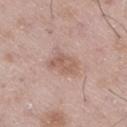Impression:
Part of a total-body skin-imaging series; this lesion was reviewed on a skin check and was not flagged for biopsy.
Background:
The total-body-photography lesion software estimated an area of roughly 7 mm² and a shape-asymmetry score of about 0.2 (0 = symmetric). It also reported an average lesion color of about L≈58 a*≈19 b*≈26 (CIELAB), a lesion–skin lightness drop of about 9, and a normalized lesion–skin contrast near 6. It also reported a border-irregularity index near 2.5/10 and internal color variation of about 3 on a 0–10 scale. The analysis additionally found an automated nevus-likeness rating near 15 out of 100 and a lesion-detection confidence of about 100/100. The lesion is on the right thigh. A male patient about 50 years old. A region of skin cropped from a whole-body photographic capture, roughly 15 mm wide. The recorded lesion diameter is about 3.5 mm. Captured under white-light illumination.